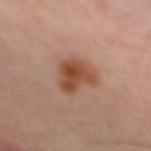biopsy status — total-body-photography surveillance lesion; no biopsy
illumination — cross-polarized illumination
subject — male, aged around 50
site — the mid back
lesion size — ≈4.5 mm
image source — ~15 mm crop, total-body skin-cancer survey